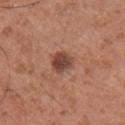Clinical summary:
The patient is a male approximately 50 years of age. The lesion's longest dimension is about 3 mm. A 15 mm close-up tile from a total-body photography series done for melanoma screening. Automated image analysis of the tile measured a footprint of about 5.5 mm², an eccentricity of roughly 0.45, and a shape-asymmetry score of about 0.2 (0 = symmetric). And it measured an average lesion color of about L≈44 a*≈22 b*≈27 (CIELAB) and roughly 13 lightness units darker than nearby skin. It also reported a nevus-likeness score of about 35/100 and a lesion-detection confidence of about 100/100. The lesion is located on the right upper arm. This is a white-light tile.